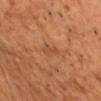This lesion was catalogued during total-body skin photography and was not selected for biopsy.
This image is a 15 mm lesion crop taken from a total-body photograph.
A male subject aged around 50.
The lesion is on the head or neck.